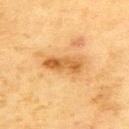Q: Was a biopsy performed?
A: catalogued during a skin exam; not biopsied
Q: Lesion location?
A: the upper back
Q: What kind of image is this?
A: ~15 mm crop, total-body skin-cancer survey
Q: How large is the lesion?
A: ~5 mm (longest diameter)
Q: Who is the patient?
A: female, aged approximately 55
Q: How was the tile lit?
A: cross-polarized